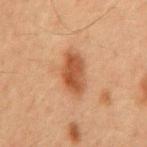Impression:
Recorded during total-body skin imaging; not selected for excision or biopsy.
Context:
A male subject, in their mid- to late 60s. The tile uses cross-polarized illumination. From the mid back. Approximately 5.5 mm at its widest. Cropped from a total-body skin-imaging series; the visible field is about 15 mm.